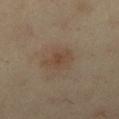Clinical impression: Captured during whole-body skin photography for melanoma surveillance; the lesion was not biopsied. Image and clinical context: Approximately 3.5 mm at its widest. From the right lower leg. An algorithmic analysis of the crop reported a classifier nevus-likeness of about 15/100 and a lesion-detection confidence of about 100/100. Captured under cross-polarized illumination. A 15 mm close-up tile from a total-body photography series done for melanoma screening. A female patient aged around 40.